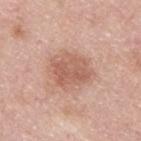Impression:
Part of a total-body skin-imaging series; this lesion was reviewed on a skin check and was not flagged for biopsy.
Image and clinical context:
The lesion-visualizer software estimated a footprint of about 14 mm², an outline eccentricity of about 0.6 (0 = round, 1 = elongated), and a symmetry-axis asymmetry near 0.25. The analysis additionally found a lesion color around L≈59 a*≈23 b*≈28 in CIELAB, about 10 CIELAB-L* units darker than the surrounding skin, and a normalized lesion–skin contrast near 6.5. It also reported border irregularity of about 2.5 on a 0–10 scale, internal color variation of about 3.5 on a 0–10 scale, and peripheral color asymmetry of about 1. The software also gave a classifier nevus-likeness of about 30/100 and lesion-presence confidence of about 100/100. The lesion is on the upper back. The recorded lesion diameter is about 4.5 mm. A male patient about 45 years old. Cropped from a whole-body photographic skin survey; the tile spans about 15 mm.